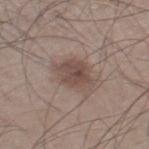Findings:
- follow-up — no biopsy performed (imaged during a skin exam)
- acquisition — 15 mm crop, total-body photography
- illumination — white-light
- subject — male, aged approximately 60
- size — about 4.5 mm
- location — the left lower leg